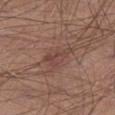Q: Was a biopsy performed?
A: no biopsy performed (imaged during a skin exam)
Q: What kind of image is this?
A: 15 mm crop, total-body photography
Q: Where on the body is the lesion?
A: the left thigh
Q: Illumination type?
A: white-light illumination
Q: Patient demographics?
A: male, aged 23 to 27